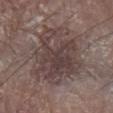<tbp_lesion>
<biopsy_status>not biopsied; imaged during a skin examination</biopsy_status>
<patient>
  <sex>male</sex>
  <age_approx>80</age_approx>
</patient>
<image>
  <source>total-body photography crop</source>
  <field_of_view_mm>15</field_of_view_mm>
</image>
<site>arm</site>
<automated_metrics>
  <cielab_L>41</cielab_L>
  <cielab_a>14</cielab_a>
  <cielab_b>17</cielab_b>
  <vs_skin_darker_L>9.0</vs_skin_darker_L>
  <vs_skin_contrast_norm>7.5</vs_skin_contrast_norm>
</automated_metrics>
<lesion_size>
  <long_diameter_mm_approx>9.0</long_diameter_mm_approx>
</lesion_size>
</tbp_lesion>